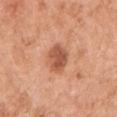<tbp_lesion>
  <biopsy_status>not biopsied; imaged during a skin examination</biopsy_status>
  <site>right upper arm</site>
  <patient>
    <sex>female</sex>
    <age_approx>50</age_approx>
  </patient>
  <image>
    <source>total-body photography crop</source>
    <field_of_view_mm>15</field_of_view_mm>
  </image>
  <lighting>white-light</lighting>
  <lesion_size>
    <long_diameter_mm_approx>4.0</long_diameter_mm_approx>
  </lesion_size>
</tbp_lesion>